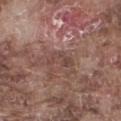Clinical impression:
Imaged during a routine full-body skin examination; the lesion was not biopsied and no histopathology is available.
Context:
The patient is a male aged 73–77. Automated image analysis of the tile measured an outline eccentricity of about 0.85 (0 = round, 1 = elongated) and two-axis asymmetry of about 0.5. And it measured a mean CIELAB color near L≈44 a*≈19 b*≈22 and about 7 CIELAB-L* units darker than the surrounding skin. And it measured an automated nevus-likeness rating near 0 out of 100 and a detector confidence of about 90 out of 100 that the crop contains a lesion. Imaged with white-light lighting. Located on the leg. A region of skin cropped from a whole-body photographic capture, roughly 15 mm wide. Approximately 3.5 mm at its widest.